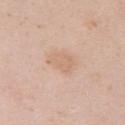Background: The total-body-photography lesion software estimated an average lesion color of about L≈67 a*≈18 b*≈31 (CIELAB) and a lesion–skin lightness drop of about 6. And it measured a border-irregularity index near 2.5/10, a within-lesion color-variation index near 1.5/10, and radial color variation of about 0.5. Measured at roughly 3 mm in maximum diameter. The lesion is located on the right upper arm. A female patient aged approximately 30. A lesion tile, about 15 mm wide, cut from a 3D total-body photograph.